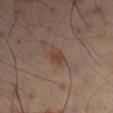The lesion was photographed on a routine skin check and not biopsied; there is no pathology result. A male subject, aged 48–52. This is a cross-polarized tile. This image is a 15 mm lesion crop taken from a total-body photograph. The lesion is on the left arm.